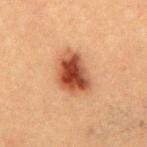Clinical impression:
No biopsy was performed on this lesion — it was imaged during a full skin examination and was not determined to be concerning.